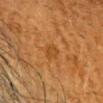{
  "biopsy_status": "not biopsied; imaged during a skin examination",
  "lighting": "cross-polarized",
  "site": "head or neck",
  "patient": {
    "sex": "male",
    "age_approx": 60
  },
  "automated_metrics": {
    "vs_skin_darker_L": 5.0,
    "vs_skin_contrast_norm": 5.0,
    "lesion_detection_confidence_0_100": 100
  },
  "image": {
    "source": "total-body photography crop",
    "field_of_view_mm": 15
  }
}